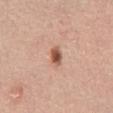Impression:
The lesion was tiled from a total-body skin photograph and was not biopsied.
Acquisition and patient details:
The lesion is located on the abdomen. A 15 mm close-up extracted from a 3D total-body photography capture. Imaged with white-light lighting. The patient is a female about 60 years old. About 2.5 mm across.